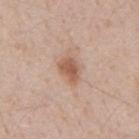No biopsy was performed on this lesion — it was imaged during a full skin examination and was not determined to be concerning. A male patient approximately 50 years of age. On the mid back. Approximately 3.5 mm at its widest. The total-body-photography lesion software estimated a mean CIELAB color near L≈57 a*≈20 b*≈29. The software also gave a border-irregularity index near 2.5/10 and a color-variation rating of about 2/10. The analysis additionally found a nevus-likeness score of about 90/100. Cropped from a whole-body photographic skin survey; the tile spans about 15 mm.